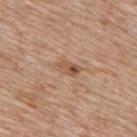follow-up: total-body-photography surveillance lesion; no biopsy
automated lesion analysis: an area of roughly 3.5 mm², an eccentricity of roughly 0.85, and a shape-asymmetry score of about 0.25 (0 = symmetric); a border-irregularity rating of about 2.5/10, a within-lesion color-variation index near 9/10, and peripheral color asymmetry of about 4; a classifier nevus-likeness of about 0/100 and a lesion-detection confidence of about 100/100
image: ~15 mm tile from a whole-body skin photo
tile lighting: white-light illumination
anatomic site: the mid back
subject: male, roughly 60 years of age
lesion diameter: ~3 mm (longest diameter)